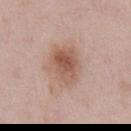Q: Was a biopsy performed?
A: catalogued during a skin exam; not biopsied
Q: How was this image acquired?
A: ~15 mm crop, total-body skin-cancer survey
Q: Who is the patient?
A: male, about 55 years old
Q: Lesion location?
A: the chest
Q: Lesion size?
A: about 5 mm